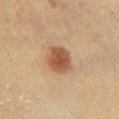Q: Was a biopsy performed?
A: total-body-photography surveillance lesion; no biopsy
Q: Where on the body is the lesion?
A: the right lower leg
Q: What is the imaging modality?
A: total-body-photography crop, ~15 mm field of view
Q: Who is the patient?
A: male, in their mid-60s
Q: What is the lesion's diameter?
A: ≈3.5 mm
Q: What did automated image analysis measure?
A: a lesion area of about 8.5 mm², an eccentricity of roughly 0.5, and a symmetry-axis asymmetry near 0.15; an average lesion color of about L≈41 a*≈18 b*≈27 (CIELAB), roughly 11 lightness units darker than nearby skin, and a lesion-to-skin contrast of about 9 (normalized; higher = more distinct); a border-irregularity index near 1.5/10 and peripheral color asymmetry of about 1; an automated nevus-likeness rating near 100 out of 100